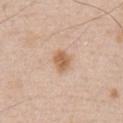| field | value |
|---|---|
| notes | no biopsy performed (imaged during a skin exam) |
| tile lighting | white-light illumination |
| TBP lesion metrics | an area of roughly 4.5 mm² and an eccentricity of roughly 0.6; a border-irregularity index near 2/10, a color-variation rating of about 2/10, and radial color variation of about 1; a detector confidence of about 100 out of 100 that the crop contains a lesion |
| lesion diameter | about 2.5 mm |
| patient | male, aged 48 to 52 |
| site | the chest |
| acquisition | ~15 mm crop, total-body skin-cancer survey |